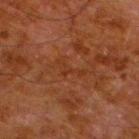Imaged during a routine full-body skin examination; the lesion was not biopsied and no histopathology is available. The lesion-visualizer software estimated a lesion color around L≈27 a*≈21 b*≈28 in CIELAB, a lesion–skin lightness drop of about 4, and a normalized lesion–skin contrast near 4.5. The software also gave a border-irregularity index near 8/10, internal color variation of about 0 on a 0–10 scale, and radial color variation of about 0. The analysis additionally found a nevus-likeness score of about 0/100 and lesion-presence confidence of about 75/100. From the leg. The patient is a male aged approximately 80. This is a cross-polarized tile. This image is a 15 mm lesion crop taken from a total-body photograph.